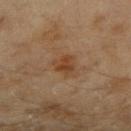Case summary:
• biopsy status — catalogued during a skin exam; not biopsied
• subject — female, in their 60s
• body site — the arm
• size — ~2.5 mm (longest diameter)
• image source — ~15 mm tile from a whole-body skin photo
• lighting — cross-polarized illumination